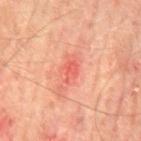Q: Was this lesion biopsied?
A: total-body-photography surveillance lesion; no biopsy
Q: Patient demographics?
A: male, about 65 years old
Q: What is the anatomic site?
A: the mid back
Q: How was this image acquired?
A: total-body-photography crop, ~15 mm field of view
Q: Illumination type?
A: cross-polarized